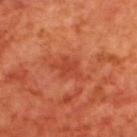No biopsy was performed on this lesion — it was imaged during a full skin examination and was not determined to be concerning.
A 15 mm crop from a total-body photograph taken for skin-cancer surveillance.
The subject is a male roughly 70 years of age.
From the upper back.